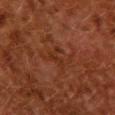Assessment:
Imaged during a routine full-body skin examination; the lesion was not biopsied and no histopathology is available.
Acquisition and patient details:
The total-body-photography lesion software estimated an average lesion color of about L≈24 a*≈21 b*≈26 (CIELAB). The software also gave an automated nevus-likeness rating near 0 out of 100 and a lesion-detection confidence of about 95/100. A 15 mm close-up tile from a total-body photography series done for melanoma screening. This is a cross-polarized tile. Approximately 3 mm at its widest. A female subject aged around 50. From the upper back.